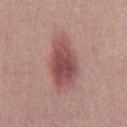Case summary:
- imaging modality · ~15 mm crop, total-body skin-cancer survey
- patient · male, aged 28–32
- automated lesion analysis · a footprint of about 17 mm², an outline eccentricity of about 0.85 (0 = round, 1 = elongated), and a shape-asymmetry score of about 0.2 (0 = symmetric); a lesion color around L≈50 a*≈25 b*≈21 in CIELAB, about 13 CIELAB-L* units darker than the surrounding skin, and a normalized lesion–skin contrast near 9
- tile lighting · white-light illumination
- lesion diameter · ~6.5 mm (longest diameter)